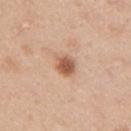No biopsy was performed on this lesion — it was imaged during a full skin examination and was not determined to be concerning.
The lesion is located on the arm.
Automated tile analysis of the lesion measured a footprint of about 5 mm² and an outline eccentricity of about 0.55 (0 = round, 1 = elongated).
The subject is a female aged around 40.
This image is a 15 mm lesion crop taken from a total-body photograph.